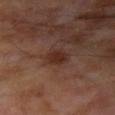Clinical impression: The lesion was photographed on a routine skin check and not biopsied; there is no pathology result. Acquisition and patient details: This is a cross-polarized tile. The total-body-photography lesion software estimated a lesion area of about 5 mm² and an outline eccentricity of about 0.7 (0 = round, 1 = elongated). The analysis additionally found a border-irregularity rating of about 1.5/10 and a peripheral color-asymmetry measure near 0.5. The analysis additionally found an automated nevus-likeness rating near 55 out of 100 and a detector confidence of about 100 out of 100 that the crop contains a lesion. A 15 mm crop from a total-body photograph taken for skin-cancer surveillance. From the left forearm. A male patient roughly 70 years of age.